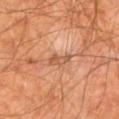Q: Is there a histopathology result?
A: no biopsy performed (imaged during a skin exam)
Q: Who is the patient?
A: male, in their mid- to late 60s
Q: What is the anatomic site?
A: the left lower leg
Q: What kind of image is this?
A: ~15 mm tile from a whole-body skin photo
Q: Illumination type?
A: cross-polarized